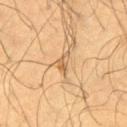Clinical impression: Recorded during total-body skin imaging; not selected for excision or biopsy. Clinical summary: The tile uses cross-polarized illumination. An algorithmic analysis of the crop reported an eccentricity of roughly 0.85 and two-axis asymmetry of about 0.45. The analysis additionally found a color-variation rating of about 3.5/10 and peripheral color asymmetry of about 1. A male patient in their mid-50s. On the left thigh. Longest diameter approximately 3 mm. A 15 mm close-up extracted from a 3D total-body photography capture.